A 15 mm crop from a total-body photograph taken for skin-cancer surveillance, on the chest, the subject is a male aged 43–47.
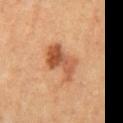| key | value |
|---|---|
| lesion size | about 5 mm |
| diagnosis | a dysplastic (Clark) nevus |